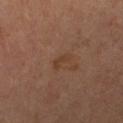Findings:
• follow-up — imaged on a skin check; not biopsied
• body site — the leg
• patient — female, aged 63–67
• acquisition — 15 mm crop, total-body photography